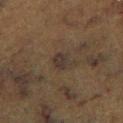follow-up: catalogued during a skin exam; not biopsied | diameter: about 2.5 mm | tile lighting: cross-polarized illumination | site: the right lower leg | imaging modality: ~15 mm crop, total-body skin-cancer survey | subject: male, in their mid-70s | automated metrics: an area of roughly 4.5 mm², an outline eccentricity of about 0.65 (0 = round, 1 = elongated), and a symmetry-axis asymmetry near 0.3; a nevus-likeness score of about 0/100.